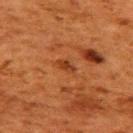  biopsy_status: not biopsied; imaged during a skin examination
  image:
    source: total-body photography crop
    field_of_view_mm: 15
  patient:
    sex: female
    age_approx: 50
  site: upper back